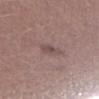workup — total-body-photography surveillance lesion; no biopsy.